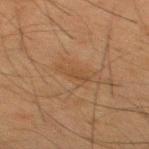workup: catalogued during a skin exam; not biopsied | image source: ~15 mm tile from a whole-body skin photo | location: the mid back | diameter: ≈3 mm | subject: male, in their mid- to late 30s | illumination: cross-polarized illumination.